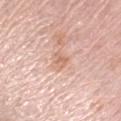follow-up=no biopsy performed (imaged during a skin exam)
image=total-body-photography crop, ~15 mm field of view
lesion size=≈2.5 mm
location=the arm
subject=female, approximately 65 years of age